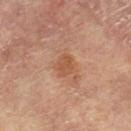The lesion was tiled from a total-body skin photograph and was not biopsied. The recorded lesion diameter is about 2.5 mm. This image is a 15 mm lesion crop taken from a total-body photograph. The lesion is located on the left lower leg. A female subject, roughly 75 years of age.